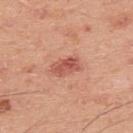image source: total-body-photography crop, ~15 mm field of view; TBP lesion metrics: a footprint of about 6 mm², an outline eccentricity of about 0.85 (0 = round, 1 = elongated), and a shape-asymmetry score of about 0.15 (0 = symmetric); anatomic site: the upper back; subject: male, about 45 years old.